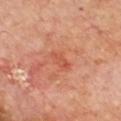notes: catalogued during a skin exam; not biopsied | tile lighting: cross-polarized illumination | lesion diameter: ≈3 mm | site: the front of the torso | TBP lesion metrics: an outline eccentricity of about 0.9 (0 = round, 1 = elongated) and a symmetry-axis asymmetry near 0.25; about 7 CIELAB-L* units darker than the surrounding skin and a normalized lesion–skin contrast near 5; a classifier nevus-likeness of about 0/100 and a detector confidence of about 100 out of 100 that the crop contains a lesion | imaging modality: 15 mm crop, total-body photography | patient: male, aged around 70.